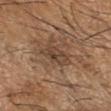This lesion was catalogued during total-body skin photography and was not selected for biopsy. A 15 mm crop from a total-body photograph taken for skin-cancer surveillance. A male subject aged 63–67. The recorded lesion diameter is about 4 mm. An algorithmic analysis of the crop reported a shape eccentricity near 0.8. The analysis additionally found border irregularity of about 3.5 on a 0–10 scale, a within-lesion color-variation index near 4.5/10, and radial color variation of about 1.5. On the right forearm. The tile uses cross-polarized illumination.